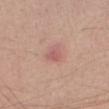| field | value |
|---|---|
| acquisition | total-body-photography crop, ~15 mm field of view |
| location | the right forearm |
| lighting | white-light illumination |
| size | ~2.5 mm (longest diameter) |
| patient | male, about 45 years old |